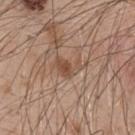Findings:
* notes: imaged on a skin check; not biopsied
* imaging modality: ~15 mm tile from a whole-body skin photo
* automated metrics: a mean CIELAB color near L≈50 a*≈19 b*≈29, about 8 CIELAB-L* units darker than the surrounding skin, and a lesion-to-skin contrast of about 6.5 (normalized; higher = more distinct); border irregularity of about 5.5 on a 0–10 scale, a color-variation rating of about 2/10, and a peripheral color-asymmetry measure near 0.5; an automated nevus-likeness rating near 15 out of 100
* illumination: white-light
* body site: the upper back
* lesion size: ~3.5 mm (longest diameter)
* patient: male, aged approximately 50